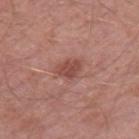Assessment: The lesion was tiled from a total-body skin photograph and was not biopsied. Acquisition and patient details: This image is a 15 mm lesion crop taken from a total-body photograph. A male subject, approximately 60 years of age. Measured at roughly 3 mm in maximum diameter. Located on the leg. This is a white-light tile. Automated tile analysis of the lesion measured an area of roughly 5.5 mm², an eccentricity of roughly 0.7, and a shape-asymmetry score of about 0.3 (0 = symmetric). The software also gave a lesion color around L≈48 a*≈25 b*≈25 in CIELAB, roughly 10 lightness units darker than nearby skin, and a lesion-to-skin contrast of about 7 (normalized; higher = more distinct).